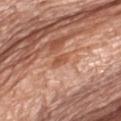Acquisition and patient details: A 15 mm close-up tile from a total-body photography series done for melanoma screening. The subject is a female aged approximately 75. Longest diameter approximately 3 mm. The lesion is located on the left thigh. Imaged with white-light lighting. An algorithmic analysis of the crop reported a shape eccentricity near 0.9 and a symmetry-axis asymmetry near 0.25. The analysis additionally found a mean CIELAB color near L≈55 a*≈25 b*≈35. It also reported an automated nevus-likeness rating near 0 out of 100.